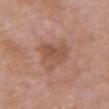{"image": {"source": "total-body photography crop", "field_of_view_mm": 15}, "patient": {"sex": "female", "age_approx": 70}, "lighting": "white-light", "site": "chest", "lesion_size": {"long_diameter_mm_approx": 4.0}}